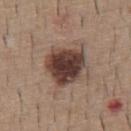  biopsy_status: not biopsied; imaged during a skin examination
  site: front of the torso
  patient:
    sex: male
    age_approx: 60
  lighting: white-light
  image:
    source: total-body photography crop
    field_of_view_mm: 15
  lesion_size:
    long_diameter_mm_approx: 5.0
  automated_metrics:
    area_mm2_approx: 17.0
    eccentricity: 0.45
    shape_asymmetry: 0.25